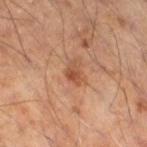{
  "biopsy_status": "not biopsied; imaged during a skin examination",
  "lesion_size": {
    "long_diameter_mm_approx": 2.5
  },
  "automated_metrics": {
    "area_mm2_approx": 3.0
  },
  "image": {
    "source": "total-body photography crop",
    "field_of_view_mm": 15
  },
  "site": "right thigh",
  "lighting": "cross-polarized",
  "patient": {
    "sex": "male",
    "age_approx": 55
  }
}